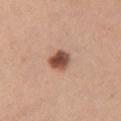Located on the chest. A female patient, in their 30s. Measured at roughly 3 mm in maximum diameter. Captured under white-light illumination. A 15 mm crop from a total-body photograph taken for skin-cancer surveillance.